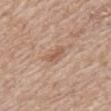biopsy status = total-body-photography surveillance lesion; no biopsy
body site = the arm
illumination = white-light
size = ≈2.5 mm
image source = ~15 mm crop, total-body skin-cancer survey
patient = male, in their 70s